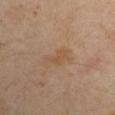Clinical impression: Captured during whole-body skin photography for melanoma surveillance; the lesion was not biopsied. Clinical summary: The lesion is located on the arm. A male patient aged around 40. Cropped from a total-body skin-imaging series; the visible field is about 15 mm.